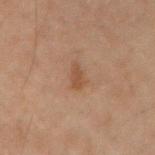<lesion>
<biopsy_status>not biopsied; imaged during a skin examination</biopsy_status>
<lighting>cross-polarized</lighting>
<image>
  <source>total-body photography crop</source>
  <field_of_view_mm>15</field_of_view_mm>
</image>
<patient>
  <sex>male</sex>
  <age_approx>70</age_approx>
</patient>
<site>right upper arm</site>
<lesion_size>
  <long_diameter_mm_approx>3.0</long_diameter_mm_approx>
</lesion_size>
</lesion>